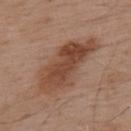| key | value |
|---|---|
| subject | male, roughly 55 years of age |
| imaging modality | 15 mm crop, total-body photography |
| location | the upper back |